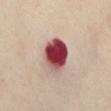No biopsy was performed on this lesion — it was imaged during a full skin examination and was not determined to be concerning.
Approximately 4.5 mm at its widest.
Captured under cross-polarized illumination.
Cropped from a total-body skin-imaging series; the visible field is about 15 mm.
Automated image analysis of the tile measured a mean CIELAB color near L≈47 a*≈32 b*≈22, a lesion–skin lightness drop of about 25, and a lesion-to-skin contrast of about 16.5 (normalized; higher = more distinct). The software also gave a color-variation rating of about 10/10 and radial color variation of about 4. It also reported a detector confidence of about 100 out of 100 that the crop contains a lesion.
The subject is a female aged around 55.
On the abdomen.